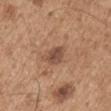Part of a total-body skin-imaging series; this lesion was reviewed on a skin check and was not flagged for biopsy. From the arm. A male subject, aged 63 to 67. About 3 mm across. A close-up tile cropped from a whole-body skin photograph, about 15 mm across.